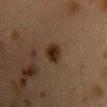Assessment: No biopsy was performed on this lesion — it was imaged during a full skin examination and was not determined to be concerning. Context: An algorithmic analysis of the crop reported a lesion color around L≈22 a*≈14 b*≈23 in CIELAB and roughly 9 lightness units darker than nearby skin. The analysis additionally found an automated nevus-likeness rating near 95 out of 100. Located on the chest. A lesion tile, about 15 mm wide, cut from a 3D total-body photograph. A female patient approximately 40 years of age. Captured under cross-polarized illumination.